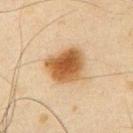Findings:
– biopsy status · total-body-photography surveillance lesion; no biopsy
– diameter · ≈4.5 mm
– patient · male, roughly 65 years of age
– anatomic site · the chest
– image · ~15 mm crop, total-body skin-cancer survey
– lighting · cross-polarized illumination
– automated lesion analysis · a lesion–skin lightness drop of about 13 and a normalized lesion–skin contrast near 11